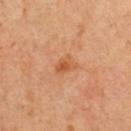Recorded during total-body skin imaging; not selected for excision or biopsy.
A male subject about 65 years old.
About 2.5 mm across.
Cropped from a total-body skin-imaging series; the visible field is about 15 mm.
The total-body-photography lesion software estimated an average lesion color of about L≈54 a*≈25 b*≈39 (CIELAB), roughly 8 lightness units darker than nearby skin, and a lesion-to-skin contrast of about 6.5 (normalized; higher = more distinct). The software also gave border irregularity of about 3 on a 0–10 scale. It also reported a lesion-detection confidence of about 100/100.
On the chest.
This is a cross-polarized tile.